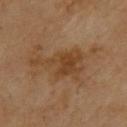The lesion was photographed on a routine skin check and not biopsied; there is no pathology result. This is a cross-polarized tile. A male patient, aged 68–72. A roughly 15 mm field-of-view crop from a total-body skin photograph. Longest diameter approximately 6.5 mm. On the upper back.